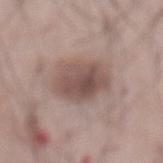No biopsy was performed on this lesion — it was imaged during a full skin examination and was not determined to be concerning.
Longest diameter approximately 5 mm.
An algorithmic analysis of the crop reported a lesion area of about 17 mm², a shape eccentricity near 0.55, and a shape-asymmetry score of about 0.15 (0 = symmetric). And it measured a border-irregularity rating of about 2/10 and internal color variation of about 5 on a 0–10 scale. It also reported a classifier nevus-likeness of about 70/100 and lesion-presence confidence of about 100/100.
Cropped from a whole-body photographic skin survey; the tile spans about 15 mm.
This is a white-light tile.
A male subject about 55 years old.
From the abdomen.